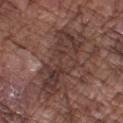Recorded during total-body skin imaging; not selected for excision or biopsy. A male patient aged approximately 75. A lesion tile, about 15 mm wide, cut from a 3D total-body photograph. Longest diameter approximately 9 mm. From the left forearm. This is a white-light tile.